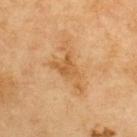Assessment:
No biopsy was performed on this lesion — it was imaged during a full skin examination and was not determined to be concerning.
Context:
A male patient, aged 68 to 72. Cropped from a whole-body photographic skin survey; the tile spans about 15 mm. On the upper back.